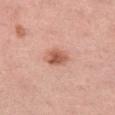Assessment:
Captured during whole-body skin photography for melanoma surveillance; the lesion was not biopsied.
Image and clinical context:
A roughly 15 mm field-of-view crop from a total-body skin photograph. The lesion is located on the left thigh. A female patient approximately 45 years of age. About 3.5 mm across. Captured under white-light illumination.